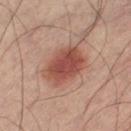The lesion was tiled from a total-body skin photograph and was not biopsied.
A male subject, approximately 40 years of age.
This image is a 15 mm lesion crop taken from a total-body photograph.
The lesion is located on the left lower leg.